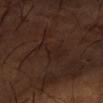Part of a total-body skin-imaging series; this lesion was reviewed on a skin check and was not flagged for biopsy. A 15 mm close-up tile from a total-body photography series done for melanoma screening. The patient is a male aged 63 to 67. The lesion is located on the left forearm. Captured under cross-polarized illumination. Approximately 4 mm at its widest.